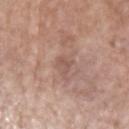Q: Was a biopsy performed?
A: imaged on a skin check; not biopsied
Q: What is the imaging modality?
A: ~15 mm tile from a whole-body skin photo
Q: What are the patient's age and sex?
A: male, in their mid- to late 70s
Q: Lesion location?
A: the left upper arm
Q: Automated lesion metrics?
A: a footprint of about 3.5 mm²; a border-irregularity rating of about 4/10, a color-variation rating of about 1.5/10, and a peripheral color-asymmetry measure near 0.5
Q: How large is the lesion?
A: about 2.5 mm